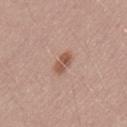Findings:
• workup: no biopsy performed (imaged during a skin exam)
• lighting: white-light
• subject: male, in their 50s
• lesion size: ≈3 mm
• image-analysis metrics: a lesion area of about 4.5 mm², an outline eccentricity of about 0.85 (0 = round, 1 = elongated), and a shape-asymmetry score of about 0.2 (0 = symmetric); a lesion–skin lightness drop of about 10 and a lesion-to-skin contrast of about 7.5 (normalized; higher = more distinct); a within-lesion color-variation index near 3.5/10 and a peripheral color-asymmetry measure near 1.5
• location: the lower back
• image: ~15 mm crop, total-body skin-cancer survey